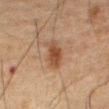notes — total-body-photography surveillance lesion; no biopsy | imaging modality — ~15 mm crop, total-body skin-cancer survey | tile lighting — cross-polarized | patient — male, about 75 years old | body site — the abdomen.